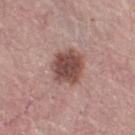Clinical impression: This lesion was catalogued during total-body skin photography and was not selected for biopsy. Image and clinical context: Captured under white-light illumination. Longest diameter approximately 4 mm. A close-up tile cropped from a whole-body skin photograph, about 15 mm across. Located on the right thigh. The subject is a female aged around 65.